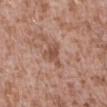Impression:
The lesion was tiled from a total-body skin photograph and was not biopsied.
Image and clinical context:
A close-up tile cropped from a whole-body skin photograph, about 15 mm across. The subject is a male in their mid-40s. This is a white-light tile. The lesion's longest dimension is about 3 mm. From the abdomen.